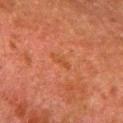Clinical impression: Part of a total-body skin-imaging series; this lesion was reviewed on a skin check and was not flagged for biopsy. Image and clinical context: The lesion-visualizer software estimated an average lesion color of about L≈39 a*≈25 b*≈33 (CIELAB), roughly 4 lightness units darker than nearby skin, and a normalized border contrast of about 5. And it measured a color-variation rating of about 0/10 and peripheral color asymmetry of about 0. About 2.5 mm across. A lesion tile, about 15 mm wide, cut from a 3D total-body photograph. A male patient aged 78 to 82. The lesion is on the left lower leg. The tile uses cross-polarized illumination.